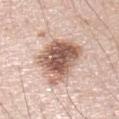Impression: Recorded during total-body skin imaging; not selected for excision or biopsy.